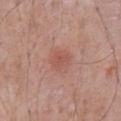Notes:
* biopsy status — total-body-photography surveillance lesion; no biopsy
* TBP lesion metrics — a footprint of about 4.5 mm² and a symmetry-axis asymmetry near 0.2; a border-irregularity rating of about 2/10 and a color-variation rating of about 2.5/10; a nevus-likeness score of about 30/100 and lesion-presence confidence of about 100/100
* size — about 2.5 mm
* imaging modality — ~15 mm tile from a whole-body skin photo
* anatomic site — the chest
* illumination — white-light
* subject — male, aged around 70